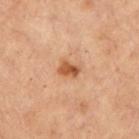notes: catalogued during a skin exam; not biopsied
anatomic site: the front of the torso
size: ≈2.5 mm
subject: female, in their mid-50s
acquisition: ~15 mm tile from a whole-body skin photo
automated metrics: a lesion area of about 4 mm², an outline eccentricity of about 0.7 (0 = round, 1 = elongated), and a symmetry-axis asymmetry near 0.25; a border-irregularity index near 2.5/10 and radial color variation of about 1.5; a detector confidence of about 100 out of 100 that the crop contains a lesion
illumination: cross-polarized